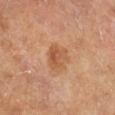subject = male, approximately 60 years of age
body site = the right lower leg
diameter = ≈3.5 mm
TBP lesion metrics = an average lesion color of about L≈52 a*≈22 b*≈34 (CIELAB), a lesion–skin lightness drop of about 8, and a normalized lesion–skin contrast near 6; an automated nevus-likeness rating near 30 out of 100 and a detector confidence of about 100 out of 100 that the crop contains a lesion
image source = total-body-photography crop, ~15 mm field of view
tile lighting = cross-polarized illumination